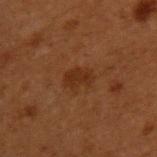Impression: Captured during whole-body skin photography for melanoma surveillance; the lesion was not biopsied. Clinical summary: This is a cross-polarized tile. A 15 mm crop from a total-body photograph taken for skin-cancer surveillance. Approximately 3 mm at its widest. The subject is a male aged approximately 50. The total-body-photography lesion software estimated a mean CIELAB color near L≈23 a*≈19 b*≈26, a lesion–skin lightness drop of about 6, and a lesion-to-skin contrast of about 7 (normalized; higher = more distinct). It also reported border irregularity of about 2 on a 0–10 scale and a color-variation rating of about 1.5/10. And it measured an automated nevus-likeness rating near 80 out of 100. From the upper back.